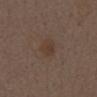{"biopsy_status": "not biopsied; imaged during a skin examination", "site": "chest", "automated_metrics": {"eccentricity": 0.8, "shape_asymmetry": 0.2, "cielab_L": 37, "cielab_a": 14, "cielab_b": 24, "vs_skin_darker_L": 5.0, "border_irregularity_0_10": 2.0, "peripheral_color_asymmetry": 0.5}, "lesion_size": {"long_diameter_mm_approx": 3.5}, "patient": {"sex": "male", "age_approx": 70}, "lighting": "white-light", "image": {"source": "total-body photography crop", "field_of_view_mm": 15}}